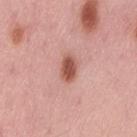This lesion was catalogued during total-body skin photography and was not selected for biopsy. On the lower back. A roughly 15 mm field-of-view crop from a total-body skin photograph. The lesion's longest dimension is about 3 mm. The tile uses white-light illumination. A female patient, approximately 50 years of age.